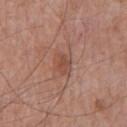<tbp_lesion>
  <image>
    <source>total-body photography crop</source>
    <field_of_view_mm>15</field_of_view_mm>
  </image>
  <patient>
    <sex>male</sex>
    <age_approx>65</age_approx>
  </patient>
  <automated_metrics>
    <cielab_L>49</cielab_L>
    <cielab_a>22</cielab_a>
    <cielab_b>28</cielab_b>
    <vs_skin_darker_L>8.0</vs_skin_darker_L>
    <vs_skin_contrast_norm>6.0</vs_skin_contrast_norm>
  </automated_metrics>
  <lighting>white-light</lighting>
  <site>chest</site>
  <lesion_size>
    <long_diameter_mm_approx>2.5</long_diameter_mm_approx>
  </lesion_size>
</tbp_lesion>